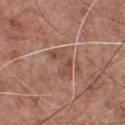Captured during whole-body skin photography for melanoma surveillance; the lesion was not biopsied.
The lesion's longest dimension is about 3.5 mm.
The lesion is located on the front of the torso.
A male subject, aged 63 to 67.
A 15 mm close-up extracted from a 3D total-body photography capture.
The tile uses white-light illumination.